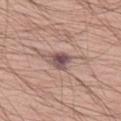biopsy status: no biopsy performed (imaged during a skin exam)
patient: male, in their mid- to late 50s
body site: the left thigh
imaging modality: total-body-photography crop, ~15 mm field of view
lesion diameter: about 2.5 mm